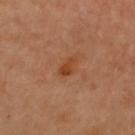The lesion was photographed on a routine skin check and not biopsied; there is no pathology result.
A region of skin cropped from a whole-body photographic capture, roughly 15 mm wide.
A female patient approximately 60 years of age.
On the front of the torso.
Longest diameter approximately 2.5 mm.
Captured under cross-polarized illumination.